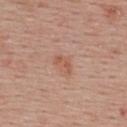Clinical impression: Imaged during a routine full-body skin examination; the lesion was not biopsied and no histopathology is available. Context: Approximately 2.5 mm at its widest. The tile uses white-light illumination. The lesion is on the upper back. A 15 mm close-up extracted from a 3D total-body photography capture. The subject is a female roughly 55 years of age.